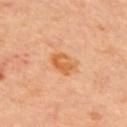{
  "biopsy_status": "not biopsied; imaged during a skin examination",
  "image": {
    "source": "total-body photography crop",
    "field_of_view_mm": 15
  },
  "patient": {
    "sex": "male",
    "age_approx": 60
  },
  "site": "back"
}